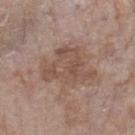Assessment:
Captured during whole-body skin photography for melanoma surveillance; the lesion was not biopsied.
Clinical summary:
Measured at roughly 6 mm in maximum diameter. Located on the right lower leg. This is a white-light tile. A 15 mm close-up tile from a total-body photography series done for melanoma screening. The patient is a female about 85 years old or older.